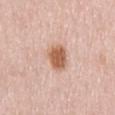Assessment:
This lesion was catalogued during total-body skin photography and was not selected for biopsy.
Background:
A 15 mm close-up tile from a total-body photography series done for melanoma screening. A female patient, aged 48 to 52. From the mid back. This is a white-light tile. Approximately 4 mm at its widest.